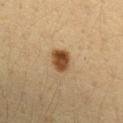The lesion was photographed on a routine skin check and not biopsied; there is no pathology result. Cropped from a total-body skin-imaging series; the visible field is about 15 mm. The lesion is located on the left forearm. A female patient, aged approximately 30.